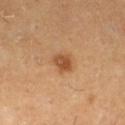Recorded during total-body skin imaging; not selected for excision or biopsy.
On the left thigh.
A lesion tile, about 15 mm wide, cut from a 3D total-body photograph.
A male subject in their 60s.
Automated tile analysis of the lesion measured about 11 CIELAB-L* units darker than the surrounding skin and a normalized border contrast of about 8. The software also gave a border-irregularity index near 1.5/10, a color-variation rating of about 4/10, and a peripheral color-asymmetry measure near 1.5. The software also gave a nevus-likeness score of about 90/100 and a detector confidence of about 100 out of 100 that the crop contains a lesion.
Measured at roughly 2.5 mm in maximum diameter.